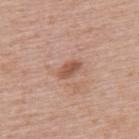Notes:
- follow-up — no biopsy performed (imaged during a skin exam)
- subject — female, roughly 60 years of age
- location — the upper back
- diameter — about 2.5 mm
- acquisition — ~15 mm crop, total-body skin-cancer survey